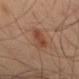Clinical impression:
The lesion was tiled from a total-body skin photograph and was not biopsied.
Background:
The tile uses cross-polarized illumination. A 15 mm crop from a total-body photograph taken for skin-cancer surveillance. A male subject aged around 50. The lesion is on the left thigh. About 5 mm across.